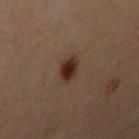Q: Was a biopsy performed?
A: catalogued during a skin exam; not biopsied
Q: What are the patient's age and sex?
A: female, approximately 30 years of age
Q: How was this image acquired?
A: ~15 mm tile from a whole-body skin photo
Q: Lesion location?
A: the left arm
Q: What is the lesion's diameter?
A: ≈2.5 mm
Q: What lighting was used for the tile?
A: cross-polarized illumination